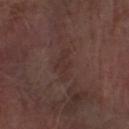<record>
<biopsy_status>not biopsied; imaged during a skin examination</biopsy_status>
<site>right forearm</site>
<patient>
  <sex>male</sex>
  <age_approx>70</age_approx>
</patient>
<image>
  <source>total-body photography crop</source>
  <field_of_view_mm>15</field_of_view_mm>
</image>
</record>